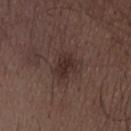No biopsy was performed on this lesion — it was imaged during a full skin examination and was not determined to be concerning.
A male subject aged around 50.
The lesion is located on the lower back.
The lesion's longest dimension is about 3.5 mm.
Cropped from a total-body skin-imaging series; the visible field is about 15 mm.
This is a white-light tile.